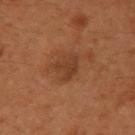Clinical summary: The recorded lesion diameter is about 3 mm. A male patient about 55 years old. The lesion is located on the left upper arm. Cropped from a total-body skin-imaging series; the visible field is about 15 mm.